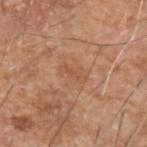Recorded during total-body skin imaging; not selected for excision or biopsy. A close-up tile cropped from a whole-body skin photograph, about 15 mm across. Located on the arm. A male patient roughly 75 years of age.